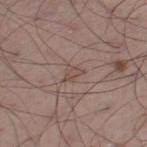{"biopsy_status": "not biopsied; imaged during a skin examination", "patient": {"sex": "male", "age_approx": 55}, "site": "left thigh", "image": {"source": "total-body photography crop", "field_of_view_mm": 15}}